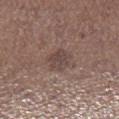Recorded during total-body skin imaging; not selected for excision or biopsy. On the leg. A male subject, approximately 75 years of age. A region of skin cropped from a whole-body photographic capture, roughly 15 mm wide. Measured at roughly 3 mm in maximum diameter. Automated image analysis of the tile measured a lesion area of about 5 mm², a shape eccentricity near 0.55, and a shape-asymmetry score of about 0.3 (0 = symmetric). It also reported an average lesion color of about L≈43 a*≈15 b*≈20 (CIELAB) and a normalized border contrast of about 6.5. The analysis additionally found a classifier nevus-likeness of about 5/100 and lesion-presence confidence of about 100/100. Captured under white-light illumination.